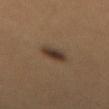anatomic site: the lower back | image: 15 mm crop, total-body photography | lesion size: ≈2.5 mm | subject: male, about 20 years old | automated lesion analysis: an area of roughly 6 mm², an outline eccentricity of about 0.4 (0 = round, 1 = elongated), and a shape-asymmetry score of about 0.15 (0 = symmetric); a border-irregularity index near 1.5/10, a within-lesion color-variation index near 6/10, and peripheral color asymmetry of about 2; a nevus-likeness score of about 100/100 and a lesion-detection confidence of about 100/100.